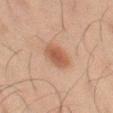{"biopsy_status": "not biopsied; imaged during a skin examination", "automated_metrics": {"area_mm2_approx": 7.5, "eccentricity": 0.8, "shape_asymmetry": 0.2, "nevus_likeness_0_100": 100, "lesion_detection_confidence_0_100": 100}, "site": "left thigh", "image": {"source": "total-body photography crop", "field_of_view_mm": 15}, "patient": {"sex": "male", "age_approx": 45}}